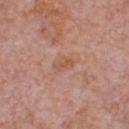| field | value |
|---|---|
| imaging modality | 15 mm crop, total-body photography |
| subject | male, aged around 60 |
| location | the chest |
| lighting | white-light |
| size | ~3 mm (longest diameter) |